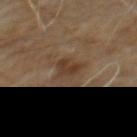No biopsy was performed on this lesion — it was imaged during a full skin examination and was not determined to be concerning.
This image is a 15 mm lesion crop taken from a total-body photograph.
Imaged with cross-polarized lighting.
Approximately 2.5 mm at its widest.
A male patient, roughly 60 years of age.
The lesion is located on the abdomen.